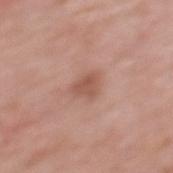follow-up: total-body-photography surveillance lesion; no biopsy
patient: female, about 40 years old
image: ~15 mm tile from a whole-body skin photo
size: ~3 mm (longest diameter)
automated metrics: an area of roughly 5 mm²; a mean CIELAB color near L≈54 a*≈23 b*≈28, a lesion–skin lightness drop of about 9, and a normalized lesion–skin contrast near 6; a color-variation rating of about 2/10 and peripheral color asymmetry of about 1
tile lighting: white-light illumination
location: the back